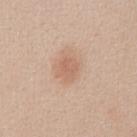illumination = white-light
lesion diameter = ~3 mm (longest diameter)
patient = female, about 20 years old
automated metrics = an area of roughly 5.5 mm², an eccentricity of roughly 0.55, and a symmetry-axis asymmetry near 0.15; an average lesion color of about L≈62 a*≈20 b*≈30 (CIELAB) and a lesion–skin lightness drop of about 8
anatomic site = the abdomen
image source = total-body-photography crop, ~15 mm field of view